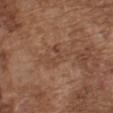follow-up — no biopsy performed (imaged during a skin exam)
tile lighting — white-light
automated lesion analysis — a footprint of about 4.5 mm², an outline eccentricity of about 0.7 (0 = round, 1 = elongated), and a symmetry-axis asymmetry near 0.55; an average lesion color of about L≈44 a*≈19 b*≈29 (CIELAB) and a normalized lesion–skin contrast near 4.5; border irregularity of about 6.5 on a 0–10 scale, internal color variation of about 0 on a 0–10 scale, and peripheral color asymmetry of about 0
size — about 3 mm
image source — ~15 mm tile from a whole-body skin photo
patient — male, approximately 75 years of age
site — the chest